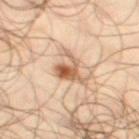Clinical impression:
Part of a total-body skin-imaging series; this lesion was reviewed on a skin check and was not flagged for biopsy.
Clinical summary:
On the leg. A 15 mm crop from a total-body photograph taken for skin-cancer surveillance. A male subject, approximately 65 years of age. About 5 mm across. The lesion-visualizer software estimated a shape eccentricity near 0.75 and a symmetry-axis asymmetry near 0.45. The software also gave a mean CIELAB color near L≈57 a*≈19 b*≈32, about 14 CIELAB-L* units darker than the surrounding skin, and a lesion-to-skin contrast of about 9 (normalized; higher = more distinct). The software also gave a nevus-likeness score of about 90/100 and a lesion-detection confidence of about 100/100.